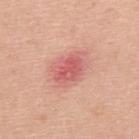Located on the mid back.
The lesion-visualizer software estimated a footprint of about 8 mm², an outline eccentricity of about 0.7 (0 = round, 1 = elongated), and a symmetry-axis asymmetry near 0.2. And it measured a lesion-detection confidence of about 100/100.
The subject is a male aged 43–47.
This is a white-light tile.
Cropped from a whole-body photographic skin survey; the tile spans about 15 mm.
Longest diameter approximately 3.5 mm.